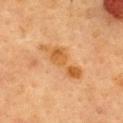notes = catalogued during a skin exam; not biopsied
tile lighting = cross-polarized
TBP lesion metrics = a border-irregularity rating of about 5.5/10, a color-variation rating of about 3.5/10, and radial color variation of about 1
patient = female, aged 58–62
imaging modality = total-body-photography crop, ~15 mm field of view
location = the upper back